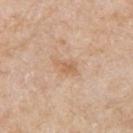The lesion was tiled from a total-body skin photograph and was not biopsied. Automated image analysis of the tile measured a footprint of about 3.5 mm², an eccentricity of roughly 0.8, and two-axis asymmetry of about 0.35. And it measured a color-variation rating of about 1.5/10. From the right upper arm. A 15 mm close-up extracted from a 3D total-body photography capture. The patient is a male in their mid- to late 60s.